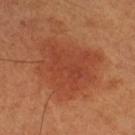Q: Is there a histopathology result?
A: imaged on a skin check; not biopsied
Q: How was this image acquired?
A: ~15 mm crop, total-body skin-cancer survey
Q: Automated lesion metrics?
A: border irregularity of about 3.5 on a 0–10 scale and a color-variation rating of about 3/10; a lesion-detection confidence of about 100/100
Q: Who is the patient?
A: male, about 50 years old
Q: Lesion size?
A: ≈7.5 mm
Q: What is the anatomic site?
A: the right thigh